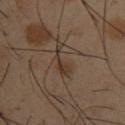lighting: cross-polarized
lesion_size:
  long_diameter_mm_approx: 4.0
image:
  source: total-body photography crop
  field_of_view_mm: 15
automated_metrics:
  area_mm2_approx: 4.5
  eccentricity: 0.9
  nevus_likeness_0_100: 0
  lesion_detection_confidence_0_100: 95
patient:
  sex: male
  age_approx: 40
site: upper back